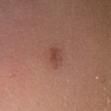workup — catalogued during a skin exam; not biopsied | image — ~15 mm crop, total-body skin-cancer survey | lighting — cross-polarized | subject — female, about 55 years old | diameter — ~2 mm (longest diameter) | body site — the leg.